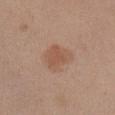notes=imaged on a skin check; not biopsied | patient=male, about 55 years old | automated metrics=an area of roughly 8.5 mm², an eccentricity of roughly 0.6, and a symmetry-axis asymmetry near 0.25; a border-irregularity index near 2.5/10, a within-lesion color-variation index near 2.5/10, and radial color variation of about 0.5 | image source=15 mm crop, total-body photography | size=~3.5 mm (longest diameter) | location=the arm.